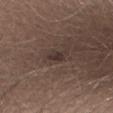<case>
<biopsy_status>not biopsied; imaged during a skin examination</biopsy_status>
<image>
  <source>total-body photography crop</source>
  <field_of_view_mm>15</field_of_view_mm>
</image>
<lighting>white-light</lighting>
<patient>
  <sex>male</sex>
  <age_approx>25</age_approx>
</patient>
<site>lower back</site>
</case>